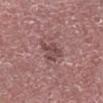The subject is a male in their mid- to late 70s. Longest diameter approximately 3 mm. A close-up tile cropped from a whole-body skin photograph, about 15 mm across. The lesion is located on the right lower leg. Imaged with white-light lighting.